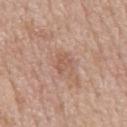No biopsy was performed on this lesion — it was imaged during a full skin examination and was not determined to be concerning.
The lesion is located on the abdomen.
The subject is a male approximately 65 years of age.
A close-up tile cropped from a whole-body skin photograph, about 15 mm across.